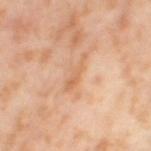Notes:
• biopsy status · total-body-photography surveillance lesion; no biopsy
• location · the leg
• patient · female, in their mid- to late 50s
• image source · 15 mm crop, total-body photography